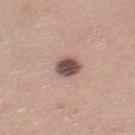This lesion was catalogued during total-body skin photography and was not selected for biopsy. This image is a 15 mm lesion crop taken from a total-body photograph. On the right lower leg. A female subject, aged 38–42.